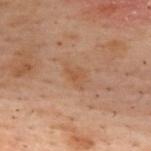| feature | finding |
|---|---|
| biopsy status | imaged on a skin check; not biopsied |
| patient | female, about 55 years old |
| imaging modality | ~15 mm crop, total-body skin-cancer survey |
| anatomic site | the upper back |